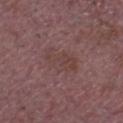Recorded during total-body skin imaging; not selected for excision or biopsy. Cropped from a total-body skin-imaging series; the visible field is about 15 mm. Imaged with white-light lighting. Measured at roughly 4.5 mm in maximum diameter. The patient is a male approximately 65 years of age. The lesion-visualizer software estimated border irregularity of about 4 on a 0–10 scale and radial color variation of about 1. The software also gave a nevus-likeness score of about 0/100 and lesion-presence confidence of about 100/100. The lesion is located on the head or neck.